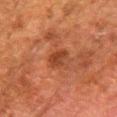| key | value |
|---|---|
| notes | catalogued during a skin exam; not biopsied |